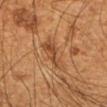No biopsy was performed on this lesion — it was imaged during a full skin examination and was not determined to be concerning.